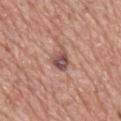workup: no biopsy performed (imaged during a skin exam); image source: ~15 mm crop, total-body skin-cancer survey; body site: the mid back; subject: male, approximately 75 years of age.